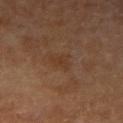Longest diameter approximately 2.5 mm. The lesion is on the right upper arm. A 15 mm close-up extracted from a 3D total-body photography capture. Automated image analysis of the tile measured a mean CIELAB color near L≈34 a*≈18 b*≈28, roughly 4 lightness units darker than nearby skin, and a normalized border contrast of about 5. It also reported a nevus-likeness score of about 0/100 and a detector confidence of about 100 out of 100 that the crop contains a lesion. The patient is a male aged 83 or older. The tile uses cross-polarized illumination.